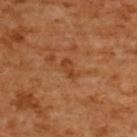Imaged during a routine full-body skin examination; the lesion was not biopsied and no histopathology is available.
Approximately 2.5 mm at its widest.
Captured under cross-polarized illumination.
A female patient in their mid- to late 50s.
The total-body-photography lesion software estimated a mean CIELAB color near L≈44 a*≈27 b*≈39, a lesion–skin lightness drop of about 8, and a lesion-to-skin contrast of about 6 (normalized; higher = more distinct).
Located on the back.
Cropped from a total-body skin-imaging series; the visible field is about 15 mm.